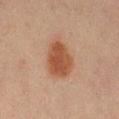Impression: Captured during whole-body skin photography for melanoma surveillance; the lesion was not biopsied.